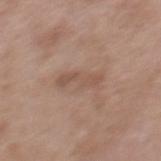A lesion tile, about 15 mm wide, cut from a 3D total-body photograph. The patient is a male aged 53 to 57. The lesion is on the mid back. The recorded lesion diameter is about 4.5 mm.